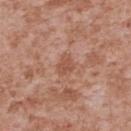notes: total-body-photography surveillance lesion; no biopsy
lesion size: about 2.5 mm
automated lesion analysis: an area of roughly 4 mm² and a shape-asymmetry score of about 0.3 (0 = symmetric); roughly 8 lightness units darker than nearby skin and a normalized lesion–skin contrast near 6; a border-irregularity rating of about 3/10, internal color variation of about 1 on a 0–10 scale, and radial color variation of about 0.5
acquisition: total-body-photography crop, ~15 mm field of view
lighting: white-light illumination
patient: male, in their mid-40s
body site: the upper back